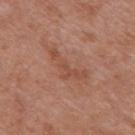Q: Was a biopsy performed?
A: imaged on a skin check; not biopsied
Q: What lighting was used for the tile?
A: white-light
Q: Where on the body is the lesion?
A: the back
Q: What are the patient's age and sex?
A: male, approximately 70 years of age
Q: How was this image acquired?
A: 15 mm crop, total-body photography